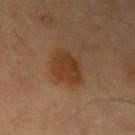TBP lesion metrics: a lesion color around L≈28 a*≈16 b*≈27 in CIELAB and a lesion–skin lightness drop of about 7; a border-irregularity rating of about 2/10, internal color variation of about 2.5 on a 0–10 scale, and a peripheral color-asymmetry measure near 1; a classifier nevus-likeness of about 85/100 and a detector confidence of about 100 out of 100 that the crop contains a lesion | patient: male, about 65 years old | size: ~4 mm (longest diameter) | anatomic site: the right upper arm | image source: ~15 mm tile from a whole-body skin photo | illumination: cross-polarized.